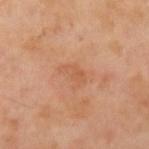Clinical impression: Recorded during total-body skin imaging; not selected for excision or biopsy. Context: The lesion is located on the left forearm. A lesion tile, about 15 mm wide, cut from a 3D total-body photograph. Automated image analysis of the tile measured a footprint of about 4.5 mm², a shape eccentricity near 0.85, and two-axis asymmetry of about 0.4. It also reported a mean CIELAB color near L≈59 a*≈24 b*≈37, about 6 CIELAB-L* units darker than the surrounding skin, and a normalized lesion–skin contrast near 4.5. And it measured a classifier nevus-likeness of about 0/100 and lesion-presence confidence of about 100/100. The recorded lesion diameter is about 3 mm. The subject is a female about 55 years old. Imaged with cross-polarized lighting.